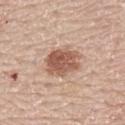The lesion is on the upper back. A close-up tile cropped from a whole-body skin photograph, about 15 mm across. Imaged with white-light lighting. The lesion's longest dimension is about 4.5 mm. The subject is a male about 60 years old.